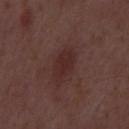Assessment: No biopsy was performed on this lesion — it was imaged during a full skin examination and was not determined to be concerning. Image and clinical context: A male patient, aged approximately 50. A 15 mm close-up tile from a total-body photography series done for melanoma screening.